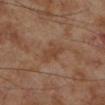| feature | finding |
|---|---|
| biopsy status | catalogued during a skin exam; not biopsied |
| body site | the left lower leg |
| patient | male, roughly 70 years of age |
| lesion size | ~4 mm (longest diameter) |
| image source | total-body-photography crop, ~15 mm field of view |